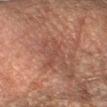Assessment: The lesion was tiled from a total-body skin photograph and was not biopsied. Clinical summary: The lesion's longest dimension is about 5 mm. This image is a 15 mm lesion crop taken from a total-body photograph. The lesion is located on the right forearm. The subject is a male aged approximately 65. This is a cross-polarized tile. Automated tile analysis of the lesion measured a lesion area of about 4.5 mm², an outline eccentricity of about 0.95 (0 = round, 1 = elongated), and a symmetry-axis asymmetry near 0.45. And it measured a lesion–skin lightness drop of about 5 and a lesion-to-skin contrast of about 5 (normalized; higher = more distinct). The analysis additionally found an automated nevus-likeness rating near 0 out of 100 and a lesion-detection confidence of about 55/100.